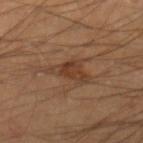| field | value |
|---|---|
| notes | total-body-photography surveillance lesion; no biopsy |
| site | the right lower leg |
| illumination | cross-polarized |
| image | 15 mm crop, total-body photography |
| patient | male, in their 40s |